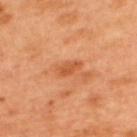No biopsy was performed on this lesion — it was imaged during a full skin examination and was not determined to be concerning.
A male subject aged 48 to 52.
Measured at roughly 3 mm in maximum diameter.
The tile uses cross-polarized illumination.
A 15 mm close-up extracted from a 3D total-body photography capture.
The lesion is on the upper back.